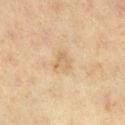The lesion was tiled from a total-body skin photograph and was not biopsied.
From the right lower leg.
A region of skin cropped from a whole-body photographic capture, roughly 15 mm wide.
The subject is a female roughly 40 years of age.